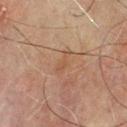Q: Was this lesion biopsied?
A: imaged on a skin check; not biopsied
Q: Who is the patient?
A: male, aged around 70
Q: Automated lesion metrics?
A: a footprint of about 3 mm² and an eccentricity of roughly 0.9; a normalized lesion–skin contrast near 4.5; peripheral color asymmetry of about 0; a nevus-likeness score of about 0/100
Q: Where on the body is the lesion?
A: the chest
Q: How large is the lesion?
A: about 3 mm
Q: How was the tile lit?
A: cross-polarized illumination
Q: What kind of image is this?
A: ~15 mm crop, total-body skin-cancer survey